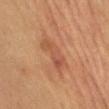| feature | finding |
|---|---|
| biopsy status | no biopsy performed (imaged during a skin exam) |
| size | ~5 mm (longest diameter) |
| lighting | cross-polarized illumination |
| body site | the chest |
| automated lesion analysis | an outline eccentricity of about 0.95 (0 = round, 1 = elongated) and a symmetry-axis asymmetry near 0.35; roughly 6 lightness units darker than nearby skin and a normalized border contrast of about 5.5; border irregularity of about 5 on a 0–10 scale and a within-lesion color-variation index near 4.5/10 |
| imaging modality | total-body-photography crop, ~15 mm field of view |
| patient | female, aged 58 to 62 |